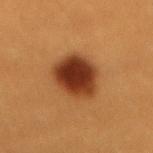  image:
    source: total-body photography crop
    field_of_view_mm: 15
  lighting: cross-polarized
  automated_metrics:
    area_mm2_approx: 17.0
    eccentricity: 0.6
    shape_asymmetry: 0.15
    border_irregularity_0_10: 1.5
    peripheral_color_asymmetry: 1.5
    nevus_likeness_0_100: 100
    lesion_detection_confidence_0_100: 100
  patient:
    sex: female
    age_approx: 30
  site: back